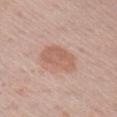  biopsy_status: not biopsied; imaged during a skin examination
  patient:
    sex: male
    age_approx: 60
  site: arm
  lesion_size:
    long_diameter_mm_approx: 4.5
  automated_metrics:
    area_mm2_approx: 11.0
    eccentricity: 0.8
    shape_asymmetry: 0.2
    peripheral_color_asymmetry: 1.0
    nevus_likeness_0_100: 60
    lesion_detection_confidence_0_100: 100
  image:
    source: total-body photography crop
    field_of_view_mm: 15